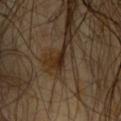Recorded during total-body skin imaging; not selected for excision or biopsy. About 1.5 mm across. A lesion tile, about 15 mm wide, cut from a 3D total-body photograph. Automated image analysis of the tile measured an automated nevus-likeness rating near 0 out of 100 and a detector confidence of about 70 out of 100 that the crop contains a lesion. A male patient, about 60 years old. The tile uses cross-polarized illumination. From the chest.